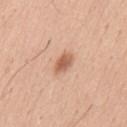Captured during whole-body skin photography for melanoma surveillance; the lesion was not biopsied. A roughly 15 mm field-of-view crop from a total-body skin photograph. On the mid back. A male patient aged 38 to 42.